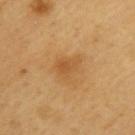Q: Was a biopsy performed?
A: catalogued during a skin exam; not biopsied
Q: Patient demographics?
A: male, about 60 years old
Q: Illumination type?
A: cross-polarized illumination
Q: Where on the body is the lesion?
A: the upper back
Q: What kind of image is this?
A: ~15 mm crop, total-body skin-cancer survey
Q: What is the lesion's diameter?
A: ≈3.5 mm